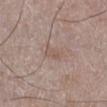This lesion was catalogued during total-body skin photography and was not selected for biopsy.